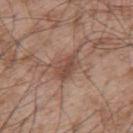Context: About 4 mm across. A close-up tile cropped from a whole-body skin photograph, about 15 mm across. From the back. Automated image analysis of the tile measured a mean CIELAB color near L≈48 a*≈19 b*≈26 and a lesion-to-skin contrast of about 6.5 (normalized; higher = more distinct). It also reported a lesion-detection confidence of about 100/100. Imaged with white-light lighting. A male patient in their mid- to late 50s.